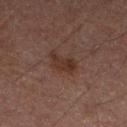follow-up = catalogued during a skin exam; not biopsied.